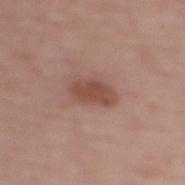Impression:
The lesion was tiled from a total-body skin photograph and was not biopsied.
Clinical summary:
An algorithmic analysis of the crop reported an area of roughly 7.5 mm², a shape eccentricity near 0.85, and a shape-asymmetry score of about 0.25 (0 = symmetric). The analysis additionally found a lesion color around L≈49 a*≈22 b*≈26 in CIELAB, about 10 CIELAB-L* units darker than the surrounding skin, and a normalized border contrast of about 7.5. Imaged with white-light lighting. The subject is a female aged 63–67. A region of skin cropped from a whole-body photographic capture, roughly 15 mm wide. On the upper back.